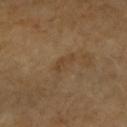Image and clinical context:
On the right forearm. This image is a 15 mm lesion crop taken from a total-body photograph. The lesion's longest dimension is about 3 mm. The patient is a female aged around 70.